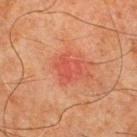  biopsy_status: not biopsied; imaged during a skin examination
  lesion_size:
    long_diameter_mm_approx: 3.0
  image:
    source: total-body photography crop
    field_of_view_mm: 15
  lighting: cross-polarized
  patient:
    sex: male
    age_approx: 65
  site: chest